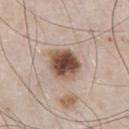notes = imaged on a skin check; not biopsied
image source = ~15 mm crop, total-body skin-cancer survey
site = the chest
lesion size = ~4 mm (longest diameter)
illumination = white-light
patient = male, roughly 55 years of age